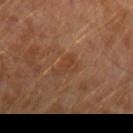No biopsy was performed on this lesion — it was imaged during a full skin examination and was not determined to be concerning. Longest diameter approximately 3 mm. A region of skin cropped from a whole-body photographic capture, roughly 15 mm wide. A male subject, aged approximately 30. Imaged with cross-polarized lighting. On the left forearm.